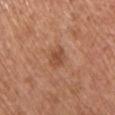Recorded during total-body skin imaging; not selected for excision or biopsy. Captured under white-light illumination. From the front of the torso. The patient is a male aged 53–57. Longest diameter approximately 2.5 mm. A region of skin cropped from a whole-body photographic capture, roughly 15 mm wide.